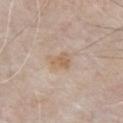biopsy status: total-body-photography surveillance lesion; no biopsy | subject: male, about 85 years old or older | acquisition: ~15 mm crop, total-body skin-cancer survey | location: the front of the torso | lesion diameter: about 3 mm.